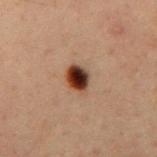  biopsy_status: not biopsied; imaged during a skin examination
  patient:
    sex: male
    age_approx: 60
  lesion_size:
    long_diameter_mm_approx: 3.5
  automated_metrics:
    area_mm2_approx: 6.5
    eccentricity: 0.65
    shape_asymmetry: 0.2
    nevus_likeness_0_100: 100
    lesion_detection_confidence_0_100: 100
  image:
    source: total-body photography crop
    field_of_view_mm: 15
  site: mid back
  lighting: cross-polarized